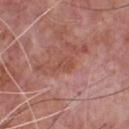Background:
A male patient, aged around 70. Imaged with white-light lighting. Longest diameter approximately 3 mm. The total-body-photography lesion software estimated a lesion–skin lightness drop of about 6 and a normalized border contrast of about 5.5. And it measured a border-irregularity index near 4/10 and internal color variation of about 1.5 on a 0–10 scale. A roughly 15 mm field-of-view crop from a total-body skin photograph. On the chest.